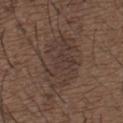  biopsy_status: not biopsied; imaged during a skin examination
  site: upper back
  lesion_size:
    long_diameter_mm_approx: 6.0
  patient:
    sex: male
    age_approx: 50
  automated_metrics:
    area_mm2_approx: 22.0
    eccentricity: 0.55
    shape_asymmetry: 0.2
    border_irregularity_0_10: 4.0
    color_variation_0_10: 3.0
    peripheral_color_asymmetry: 1.0
  lighting: white-light
  image:
    source: total-body photography crop
    field_of_view_mm: 15